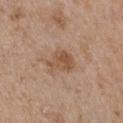Part of a total-body skin-imaging series; this lesion was reviewed on a skin check and was not flagged for biopsy.
On the chest.
A female subject, in their mid-60s.
A roughly 15 mm field-of-view crop from a total-body skin photograph.